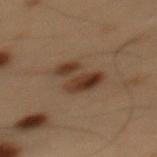The lesion was photographed on a routine skin check and not biopsied; there is no pathology result.
Captured under cross-polarized illumination.
Automated tile analysis of the lesion measured a footprint of about 9.5 mm², an eccentricity of roughly 0.8, and a symmetry-axis asymmetry near 0.5.
A male patient aged around 55.
The lesion is on the mid back.
The recorded lesion diameter is about 4.5 mm.
A 15 mm close-up extracted from a 3D total-body photography capture.